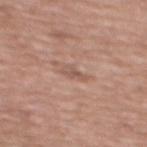The lesion was photographed on a routine skin check and not biopsied; there is no pathology result. Automated tile analysis of the lesion measured an area of roughly 3 mm² and a shape eccentricity near 0.9. The software also gave a lesion–skin lightness drop of about 8 and a lesion-to-skin contrast of about 5.5 (normalized; higher = more distinct). The analysis additionally found a within-lesion color-variation index near 1/10 and peripheral color asymmetry of about 0. And it measured a classifier nevus-likeness of about 0/100 and a lesion-detection confidence of about 100/100. The recorded lesion diameter is about 2.5 mm. Captured under white-light illumination. A female patient, aged 73–77. A lesion tile, about 15 mm wide, cut from a 3D total-body photograph. The lesion is located on the mid back.